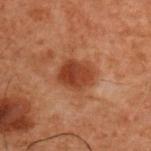Imaged during a routine full-body skin examination; the lesion was not biopsied and no histopathology is available. The lesion is located on the upper back. A male subject, aged around 50. A 15 mm crop from a total-body photograph taken for skin-cancer surveillance. About 4 mm across. Captured under cross-polarized illumination.